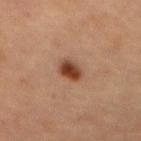notes: total-body-photography surveillance lesion; no biopsy
subject: female, roughly 60 years of age
image source: ~15 mm crop, total-body skin-cancer survey
diameter: ≈3 mm
site: the abdomen